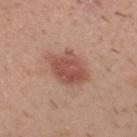The lesion was photographed on a routine skin check and not biopsied; there is no pathology result.
Measured at roughly 5.5 mm in maximum diameter.
A region of skin cropped from a whole-body photographic capture, roughly 15 mm wide.
A male subject roughly 30 years of age.
The lesion is on the head or neck.